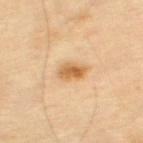Background:
The lesion's longest dimension is about 3 mm. A 15 mm close-up extracted from a 3D total-body photography capture. Located on the left thigh. A male subject aged around 70.